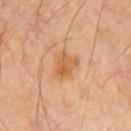Q: Is there a histopathology result?
A: no biopsy performed (imaged during a skin exam)
Q: What is the lesion's diameter?
A: ~3 mm (longest diameter)
Q: Where on the body is the lesion?
A: the chest
Q: What kind of image is this?
A: ~15 mm tile from a whole-body skin photo
Q: How was the tile lit?
A: cross-polarized
Q: Patient demographics?
A: male, roughly 60 years of age
Q: What did automated image analysis measure?
A: a mean CIELAB color near L≈58 a*≈24 b*≈40, a lesion–skin lightness drop of about 9, and a normalized border contrast of about 7.5; peripheral color asymmetry of about 1.5; a classifier nevus-likeness of about 30/100